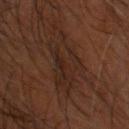Q: Is there a histopathology result?
A: no biopsy performed (imaged during a skin exam)
Q: Automated lesion metrics?
A: a footprint of about 16 mm², an outline eccentricity of about 0.75 (0 = round, 1 = elongated), and two-axis asymmetry of about 0.4; about 5 CIELAB-L* units darker than the surrounding skin; a border-irregularity index near 6/10, a color-variation rating of about 3.5/10, and peripheral color asymmetry of about 1; an automated nevus-likeness rating near 5 out of 100 and a detector confidence of about 55 out of 100 that the crop contains a lesion
Q: Lesion location?
A: the head or neck
Q: What kind of image is this?
A: 15 mm crop, total-body photography
Q: Who is the patient?
A: male, aged 58–62